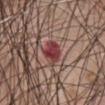workup: imaged on a skin check; not biopsied | lighting: white-light | lesion diameter: about 3 mm | image source: ~15 mm crop, total-body skin-cancer survey | location: the chest | patient: male, about 65 years old | automated metrics: a lesion area of about 7 mm², a shape eccentricity near 0.25, and a symmetry-axis asymmetry near 0.2; a lesion color around L≈41 a*≈28 b*≈21 in CIELAB, roughly 12 lightness units darker than nearby skin, and a lesion-to-skin contrast of about 9.5 (normalized; higher = more distinct); a within-lesion color-variation index near 6/10 and peripheral color asymmetry of about 2; lesion-presence confidence of about 100/100.